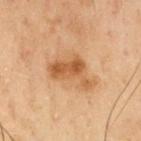Q: Was this lesion biopsied?
A: total-body-photography surveillance lesion; no biopsy
Q: Lesion size?
A: about 5.5 mm
Q: Automated lesion metrics?
A: a lesion area of about 10 mm², a shape eccentricity near 0.8, and a shape-asymmetry score of about 0.5 (0 = symmetric); a lesion color around L≈46 a*≈19 b*≈34 in CIELAB, roughly 10 lightness units darker than nearby skin, and a normalized border contrast of about 8; internal color variation of about 3.5 on a 0–10 scale
Q: Where on the body is the lesion?
A: the chest
Q: How was the tile lit?
A: cross-polarized
Q: How was this image acquired?
A: ~15 mm tile from a whole-body skin photo
Q: Who is the patient?
A: male, aged approximately 55